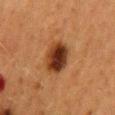The lesion was tiled from a total-body skin photograph and was not biopsied. A 15 mm crop from a total-body photograph taken for skin-cancer surveillance. Imaged with cross-polarized lighting. Measured at roughly 4 mm in maximum diameter. The subject is a female aged around 50. Located on the mid back.